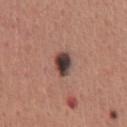workup — no biopsy performed (imaged during a skin exam) | patient — male, approximately 45 years of age | illumination — white-light | imaging modality — 15 mm crop, total-body photography | site — the chest | automated metrics — a lesion area of about 6 mm² and a shape-asymmetry score of about 0.15 (0 = symmetric); a border-irregularity index near 1.5/10, a color-variation rating of about 8/10, and peripheral color asymmetry of about 2; a classifier nevus-likeness of about 100/100 and lesion-presence confidence of about 100/100.